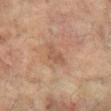  biopsy_status: not biopsied; imaged during a skin examination
  lesion_size:
    long_diameter_mm_approx: 3.0
  patient:
    sex: female
    age_approx: 80
  lighting: cross-polarized
  image:
    source: total-body photography crop
    field_of_view_mm: 15
  site: right arm
  automated_metrics:
    area_mm2_approx: 3.5
    eccentricity: 0.8
    cielab_L: 49
    cielab_a: 18
    cielab_b: 28
    vs_skin_darker_L: 7.0
    vs_skin_contrast_norm: 5.0